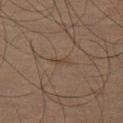Part of a total-body skin-imaging series; this lesion was reviewed on a skin check and was not flagged for biopsy.
The lesion is on the left thigh.
A male patient in their mid-60s.
A lesion tile, about 15 mm wide, cut from a 3D total-body photograph.